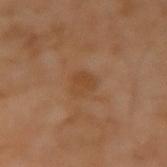Captured during whole-body skin photography for melanoma surveillance; the lesion was not biopsied.
Captured under cross-polarized illumination.
The lesion-visualizer software estimated an average lesion color of about L≈43 a*≈20 b*≈34 (CIELAB), about 6 CIELAB-L* units darker than the surrounding skin, and a normalized border contrast of about 5.5. The analysis additionally found a border-irregularity rating of about 2.5/10, a color-variation rating of about 2/10, and a peripheral color-asymmetry measure near 1.
A male patient roughly 65 years of age.
A roughly 15 mm field-of-view crop from a total-body skin photograph.
About 2.5 mm across.